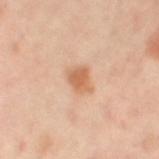Measured at roughly 2.5 mm in maximum diameter.
On the left thigh.
Cropped from a whole-body photographic skin survey; the tile spans about 15 mm.
A female patient aged 48–52.
This is a cross-polarized tile.